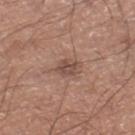The lesion was photographed on a routine skin check and not biopsied; there is no pathology result.
A 15 mm crop from a total-body photograph taken for skin-cancer surveillance.
A male patient, aged around 65.
The total-body-photography lesion software estimated an average lesion color of about L≈49 a*≈19 b*≈25 (CIELAB), about 9 CIELAB-L* units darker than the surrounding skin, and a lesion-to-skin contrast of about 7 (normalized; higher = more distinct). And it measured internal color variation of about 3.5 on a 0–10 scale and peripheral color asymmetry of about 1.5. The software also gave a classifier nevus-likeness of about 0/100.
The recorded lesion diameter is about 3 mm.
The lesion is on the right lower leg.
The tile uses white-light illumination.